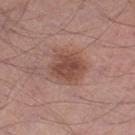workup: no biopsy performed (imaged during a skin exam)
lesion size: ≈4 mm
patient: male, aged 68 to 72
tile lighting: white-light
acquisition: ~15 mm tile from a whole-body skin photo
site: the left thigh
automated metrics: a mean CIELAB color near L≈48 a*≈21 b*≈25, a lesion–skin lightness drop of about 10, and a lesion-to-skin contrast of about 7.5 (normalized; higher = more distinct); border irregularity of about 1.5 on a 0–10 scale and a peripheral color-asymmetry measure near 1; a nevus-likeness score of about 60/100 and a detector confidence of about 100 out of 100 that the crop contains a lesion